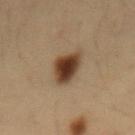workup = total-body-photography surveillance lesion; no biopsy
patient = male, aged approximately 35
tile lighting = cross-polarized
body site = the lower back
acquisition = total-body-photography crop, ~15 mm field of view